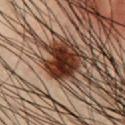follow-up = no biopsy performed (imaged during a skin exam)
image = total-body-photography crop, ~15 mm field of view
patient = male, approximately 35 years of age
TBP lesion metrics = an average lesion color of about L≈25 a*≈21 b*≈25 (CIELAB), about 18 CIELAB-L* units darker than the surrounding skin, and a normalized border contrast of about 16.5; an automated nevus-likeness rating near 90 out of 100
illumination = cross-polarized
site = the chest